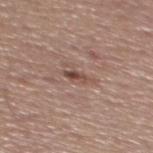automated lesion analysis: a lesion area of about 2.5 mm², an outline eccentricity of about 0.9 (0 = round, 1 = elongated), and two-axis asymmetry of about 0.3
subject: male, aged approximately 55
illumination: white-light illumination
site: the mid back
lesion size: ~2.5 mm (longest diameter)
acquisition: 15 mm crop, total-body photography